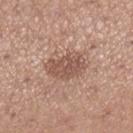Captured during whole-body skin photography for melanoma surveillance; the lesion was not biopsied.
A close-up tile cropped from a whole-body skin photograph, about 15 mm across.
A female subject in their 30s.
This is a white-light tile.
From the right lower leg.
The total-body-photography lesion software estimated a border-irregularity index near 3/10, a within-lesion color-variation index near 2.5/10, and a peripheral color-asymmetry measure near 1.